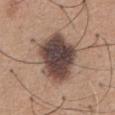Notes:
• follow-up — imaged on a skin check; not biopsied
• lesion diameter — about 7.5 mm
• image source — ~15 mm crop, total-body skin-cancer survey
• location — the chest
• subject — male, in their mid-60s
• tile lighting — white-light illumination
• automated metrics — peripheral color asymmetry of about 2